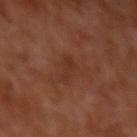notes = total-body-photography surveillance lesion; no biopsy | subject = male, about 30 years old | imaging modality = total-body-photography crop, ~15 mm field of view | anatomic site = the left upper arm | lesion size = ≈3.5 mm.